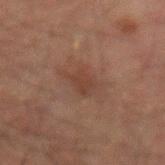The lesion was tiled from a total-body skin photograph and was not biopsied. Measured at roughly 4 mm in maximum diameter. This image is a 15 mm lesion crop taken from a total-body photograph. Located on the left forearm. Captured under cross-polarized illumination. The patient is a male roughly 50 years of age.